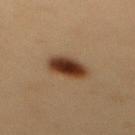Clinical impression: Part of a total-body skin-imaging series; this lesion was reviewed on a skin check and was not flagged for biopsy. Clinical summary: The subject is a female about 30 years old. The lesion is located on the upper back. A close-up tile cropped from a whole-body skin photograph, about 15 mm across.